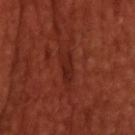The lesion was tiled from a total-body skin photograph and was not biopsied. A male patient, about 50 years old. Measured at roughly 4 mm in maximum diameter. The lesion is on the back. Cropped from a whole-body photographic skin survey; the tile spans about 15 mm.